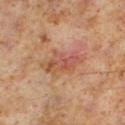{
  "biopsy_status": "not biopsied; imaged during a skin examination",
  "image": {
    "source": "total-body photography crop",
    "field_of_view_mm": 15
  },
  "automated_metrics": {
    "area_mm2_approx": 10.0,
    "eccentricity": 0.85,
    "border_irregularity_0_10": 5.0,
    "peripheral_color_asymmetry": 3.0,
    "nevus_likeness_0_100": 15,
    "lesion_detection_confidence_0_100": 100
  },
  "patient": {
    "sex": "male",
    "age_approx": 60
  },
  "lesion_size": {
    "long_diameter_mm_approx": 5.0
  },
  "site": "left lower leg"
}